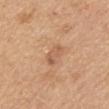biopsy status — no biopsy performed (imaged during a skin exam) | subject — female, in their mid- to late 50s | location — the mid back | acquisition — 15 mm crop, total-body photography.